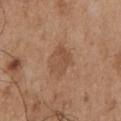Clinical impression:
Imaged during a routine full-body skin examination; the lesion was not biopsied and no histopathology is available.
Background:
On the chest. The subject is a male aged around 55. An algorithmic analysis of the crop reported a lesion area of about 7.5 mm², an eccentricity of roughly 0.65, and a symmetry-axis asymmetry near 0.25. The software also gave a border-irregularity rating of about 2.5/10 and a within-lesion color-variation index near 2.5/10. It also reported an automated nevus-likeness rating near 0 out of 100 and a lesion-detection confidence of about 100/100. A region of skin cropped from a whole-body photographic capture, roughly 15 mm wide. The lesion's longest dimension is about 3.5 mm. This is a white-light tile.